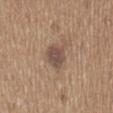Q: Was this lesion biopsied?
A: no biopsy performed (imaged during a skin exam)
Q: Lesion location?
A: the mid back
Q: What did automated image analysis measure?
A: an eccentricity of roughly 0.5 and a shape-asymmetry score of about 0.25 (0 = symmetric); a mean CIELAB color near L≈49 a*≈16 b*≈24, a lesion–skin lightness drop of about 10, and a normalized lesion–skin contrast near 8; border irregularity of about 2.5 on a 0–10 scale, internal color variation of about 2 on a 0–10 scale, and a peripheral color-asymmetry measure near 0.5
Q: What is the lesion's diameter?
A: ≈3 mm
Q: What are the patient's age and sex?
A: male, aged around 65
Q: What lighting was used for the tile?
A: white-light illumination
Q: How was this image acquired?
A: ~15 mm tile from a whole-body skin photo